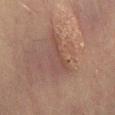Recorded during total-body skin imaging; not selected for excision or biopsy.